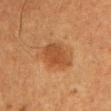Part of a total-body skin-imaging series; this lesion was reviewed on a skin check and was not flagged for biopsy.
A male subject, approximately 50 years of age.
A region of skin cropped from a whole-body photographic capture, roughly 15 mm wide.
On the left upper arm.